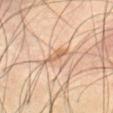Assessment:
The lesion was tiled from a total-body skin photograph and was not biopsied.
Acquisition and patient details:
Imaged with cross-polarized lighting. A male patient aged approximately 55. The lesion is located on the abdomen. The recorded lesion diameter is about 4.5 mm. A lesion tile, about 15 mm wide, cut from a 3D total-body photograph.